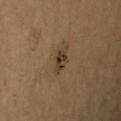Notes:
* workup — catalogued during a skin exam; not biopsied
* image source — 15 mm crop, total-body photography
* anatomic site — the right upper arm
* image-analysis metrics — roughly 10 lightness units darker than nearby skin
* tile lighting — cross-polarized illumination
* subject — male, aged 63 to 67